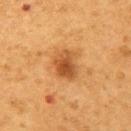patient — male, about 55 years old | acquisition — ~15 mm tile from a whole-body skin photo | automated metrics — a mean CIELAB color near L≈47 a*≈24 b*≈40, roughly 12 lightness units darker than nearby skin, and a lesion-to-skin contrast of about 8.5 (normalized; higher = more distinct); lesion-presence confidence of about 100/100 | body site — the left upper arm.